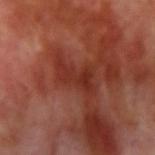workup: catalogued during a skin exam; not biopsied
automated lesion analysis: a footprint of about 8 mm² and an outline eccentricity of about 0.85 (0 = round, 1 = elongated); a classifier nevus-likeness of about 0/100 and a lesion-detection confidence of about 85/100
subject: male, aged approximately 70
size: about 5 mm
image: total-body-photography crop, ~15 mm field of view
tile lighting: cross-polarized illumination
location: the right upper arm